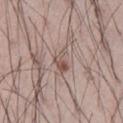| key | value |
|---|---|
| biopsy status | imaged on a skin check; not biopsied |
| anatomic site | the left thigh |
| image source | ~15 mm crop, total-body skin-cancer survey |
| subject | male, about 40 years old |